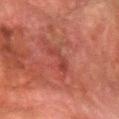{"biopsy_status": "not biopsied; imaged during a skin examination", "patient": {"sex": "male", "age_approx": 80}, "image": {"source": "total-body photography crop", "field_of_view_mm": 15}, "site": "left forearm"}